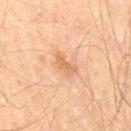– biopsy status: total-body-photography surveillance lesion; no biopsy
– acquisition: ~15 mm tile from a whole-body skin photo
– patient: male, in their 60s
– body site: the right thigh
– size: about 3.5 mm
– TBP lesion metrics: border irregularity of about 4 on a 0–10 scale, a color-variation rating of about 1/10, and peripheral color asymmetry of about 0